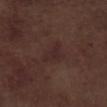follow-up: imaged on a skin check; not biopsied
lighting: white-light illumination
patient: male, approximately 70 years of age
image: 15 mm crop, total-body photography
body site: the right lower leg